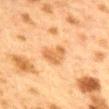Assessment: No biopsy was performed on this lesion — it was imaged during a full skin examination and was not determined to be concerning. Context: A female patient aged approximately 40. Located on the mid back. A 15 mm crop from a total-body photograph taken for skin-cancer surveillance.